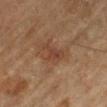A 15 mm close-up tile from a total-body photography series done for melanoma screening. The subject is a male aged 83 or older. The lesion is on the left lower leg. Captured under cross-polarized illumination.